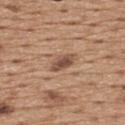Imaged during a routine full-body skin examination; the lesion was not biopsied and no histopathology is available.
A male subject, aged 63–67.
A 15 mm crop from a total-body photograph taken for skin-cancer surveillance.
The lesion is located on the upper back.